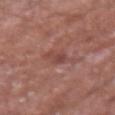Notes:
* notes — imaged on a skin check; not biopsied
* acquisition — total-body-photography crop, ~15 mm field of view
* patient — male, aged 63–67
* body site — the right forearm